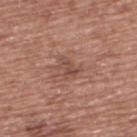Recorded during total-body skin imaging; not selected for excision or biopsy. On the upper back. The patient is a male approximately 75 years of age. This is a white-light tile. A roughly 15 mm field-of-view crop from a total-body skin photograph. Approximately 3 mm at its widest.